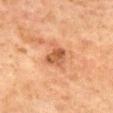Recorded during total-body skin imaging; not selected for excision or biopsy. A close-up tile cropped from a whole-body skin photograph, about 15 mm across. The lesion is on the chest. Automated tile analysis of the lesion measured a border-irregularity index near 3/10 and a peripheral color-asymmetry measure near 1.5. A female subject aged approximately 60. Captured under cross-polarized illumination. About 3 mm across.